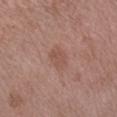Impression: Captured during whole-body skin photography for melanoma surveillance; the lesion was not biopsied. Background: A close-up tile cropped from a whole-body skin photograph, about 15 mm across. A male subject, about 50 years old. This is a white-light tile. From the left forearm. The lesion's longest dimension is about 3 mm. The total-body-photography lesion software estimated a lesion color around L≈52 a*≈20 b*≈25 in CIELAB and a lesion–skin lightness drop of about 6. And it measured a nevus-likeness score of about 0/100.